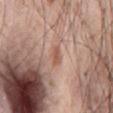Notes:
* follow-up · catalogued during a skin exam; not biopsied
* site · the abdomen
* patient · male, aged 53 to 57
* image · total-body-photography crop, ~15 mm field of view